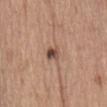Captured during whole-body skin photography for melanoma surveillance; the lesion was not biopsied. A 15 mm close-up extracted from a 3D total-body photography capture. A male patient, approximately 70 years of age. Located on the abdomen. The recorded lesion diameter is about 2.5 mm.